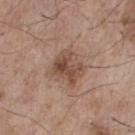  biopsy_status: not biopsied; imaged during a skin examination
  lighting: white-light
  lesion_size:
    long_diameter_mm_approx: 3.5
  site: chest
  automated_metrics:
    cielab_L: 48
    cielab_a: 19
    cielab_b: 26
    vs_skin_darker_L: 10.0
    vs_skin_contrast_norm: 7.5
    border_irregularity_0_10: 3.5
    color_variation_0_10: 5.5
    peripheral_color_asymmetry: 2.5
  patient:
    sex: male
    age_approx: 75
  image:
    source: total-body photography crop
    field_of_view_mm: 15